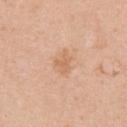Clinical impression: Part of a total-body skin-imaging series; this lesion was reviewed on a skin check and was not flagged for biopsy. Acquisition and patient details: Automated image analysis of the tile measured a lesion area of about 4 mm², an outline eccentricity of about 0.75 (0 = round, 1 = elongated), and a shape-asymmetry score of about 0.3 (0 = symmetric). The analysis additionally found an average lesion color of about L≈65 a*≈21 b*≈35 (CIELAB), a lesion–skin lightness drop of about 7, and a normalized lesion–skin contrast near 5.5. It also reported internal color variation of about 1.5 on a 0–10 scale. A female patient roughly 45 years of age. Imaged with white-light lighting. On the right upper arm. Cropped from a total-body skin-imaging series; the visible field is about 15 mm. About 3 mm across.